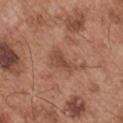| field | value |
|---|---|
| workup | imaged on a skin check; not biopsied |
| imaging modality | 15 mm crop, total-body photography |
| site | the left upper arm |
| automated lesion analysis | internal color variation of about 1.5 on a 0–10 scale; an automated nevus-likeness rating near 0 out of 100 and lesion-presence confidence of about 100/100 |
| subject | male, about 55 years old |